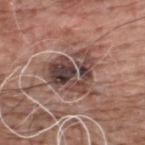Assessment: The lesion was photographed on a routine skin check and not biopsied; there is no pathology result. Clinical summary: Imaged with white-light lighting. An algorithmic analysis of the crop reported a shape eccentricity near 0.55 and two-axis asymmetry of about 0.4. It also reported a mean CIELAB color near L≈43 a*≈18 b*≈20, about 13 CIELAB-L* units darker than the surrounding skin, and a normalized lesion–skin contrast near 10. The software also gave border irregularity of about 6.5 on a 0–10 scale and radial color variation of about 3.5. And it measured a nevus-likeness score of about 0/100 and lesion-presence confidence of about 95/100. A close-up tile cropped from a whole-body skin photograph, about 15 mm across. Longest diameter approximately 6 mm. On the upper back. A male subject, about 60 years old.